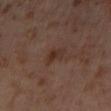This lesion was catalogued during total-body skin photography and was not selected for biopsy. On the left thigh. An algorithmic analysis of the crop reported a footprint of about 4 mm² and an outline eccentricity of about 0.7 (0 = round, 1 = elongated). And it measured a nevus-likeness score of about 5/100 and lesion-presence confidence of about 100/100. Longest diameter approximately 2.5 mm. A 15 mm crop from a total-body photograph taken for skin-cancer surveillance. This is a cross-polarized tile. The subject is a female in their mid- to late 50s.